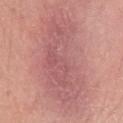Captured during whole-body skin photography for melanoma surveillance; the lesion was not biopsied.